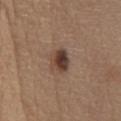Captured during whole-body skin photography for melanoma surveillance; the lesion was not biopsied. This is a white-light tile. A region of skin cropped from a whole-body photographic capture, roughly 15 mm wide. About 3.5 mm across. The lesion-visualizer software estimated an area of roughly 6.5 mm² and a shape-asymmetry score of about 0.2 (0 = symmetric). The analysis additionally found peripheral color asymmetry of about 2. The software also gave a nevus-likeness score of about 95/100. The subject is a female aged 63 to 67. The lesion is located on the abdomen.